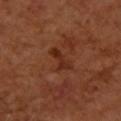biopsy_status: not biopsied; imaged during a skin examination
image:
  source: total-body photography crop
  field_of_view_mm: 15
site: right forearm
patient:
  sex: female
  age_approx: 50